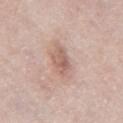Captured during whole-body skin photography for melanoma surveillance; the lesion was not biopsied.
This image is a 15 mm lesion crop taken from a total-body photograph.
An algorithmic analysis of the crop reported a mean CIELAB color near L≈60 a*≈20 b*≈25, roughly 10 lightness units darker than nearby skin, and a lesion-to-skin contrast of about 6.5 (normalized; higher = more distinct). The software also gave an automated nevus-likeness rating near 30 out of 100 and a lesion-detection confidence of about 100/100.
The recorded lesion diameter is about 3.5 mm.
A male subject, aged 73–77.
This is a white-light tile.
Located on the mid back.